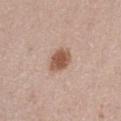No biopsy was performed on this lesion — it was imaged during a full skin examination and was not determined to be concerning. Imaged with white-light lighting. The lesion's longest dimension is about 3 mm. The patient is a female aged approximately 50. The lesion is located on the left thigh. This image is a 15 mm lesion crop taken from a total-body photograph. Automated tile analysis of the lesion measured a lesion area of about 6 mm² and an outline eccentricity of about 0.6 (0 = round, 1 = elongated).